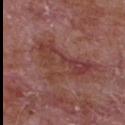{"biopsy_status": "not biopsied; imaged during a skin examination", "site": "front of the torso", "automated_metrics": {"area_mm2_approx": 19.0, "shape_asymmetry": 0.55, "border_irregularity_0_10": 7.0, "peripheral_color_asymmetry": 2.0}, "lighting": "white-light", "image": {"source": "total-body photography crop", "field_of_view_mm": 15}, "lesion_size": {"long_diameter_mm_approx": 8.0}, "patient": {"sex": "male", "age_approx": 65}}